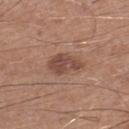workup — imaged on a skin check; not biopsied
site — the left lower leg
patient — male, aged 58 to 62
imaging modality — ~15 mm tile from a whole-body skin photo